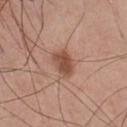Longest diameter approximately 3.5 mm. A male subject, aged around 30. Automated image analysis of the tile measured a lesion color around L≈49 a*≈23 b*≈29 in CIELAB and roughly 12 lightness units darker than nearby skin. And it measured a border-irregularity rating of about 2/10 and a color-variation rating of about 2.5/10. And it measured a nevus-likeness score of about 90/100 and lesion-presence confidence of about 100/100. From the front of the torso. Cropped from a whole-body photographic skin survey; the tile spans about 15 mm.